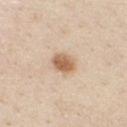Imaged during a routine full-body skin examination; the lesion was not biopsied and no histopathology is available. Measured at roughly 3 mm in maximum diameter. The lesion is located on the front of the torso. The total-body-photography lesion software estimated a lesion area of about 6 mm², a shape eccentricity near 0.65, and a symmetry-axis asymmetry near 0.2. The software also gave about 13 CIELAB-L* units darker than the surrounding skin and a normalized border contrast of about 9. The software also gave a border-irregularity index near 1.5/10 and internal color variation of about 3.5 on a 0–10 scale. The software also gave a detector confidence of about 100 out of 100 that the crop contains a lesion. The tile uses white-light illumination. The subject is a male aged 28–32. A 15 mm crop from a total-body photograph taken for skin-cancer surveillance.